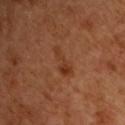A close-up tile cropped from a whole-body skin photograph, about 15 mm across.
Measured at roughly 4 mm in maximum diameter.
A male patient, about 50 years old.
From the front of the torso.